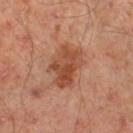workup: imaged on a skin check; not biopsied.